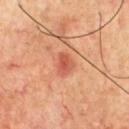The lesion was photographed on a routine skin check and not biopsied; there is no pathology result. The total-body-photography lesion software estimated an eccentricity of roughly 0.7 and a symmetry-axis asymmetry near 0.3. The patient is a male aged 68–72. Cropped from a total-body skin-imaging series; the visible field is about 15 mm. Approximately 2.5 mm at its widest. On the chest.